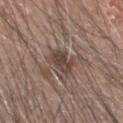| key | value |
|---|---|
| follow-up | total-body-photography surveillance lesion; no biopsy |
| subject | male, in their mid-40s |
| TBP lesion metrics | a lesion area of about 6.5 mm², an outline eccentricity of about 0.85 (0 = round, 1 = elongated), and a shape-asymmetry score of about 0.3 (0 = symmetric); a nevus-likeness score of about 15/100 and a detector confidence of about 90 out of 100 that the crop contains a lesion |
| image source | ~15 mm crop, total-body skin-cancer survey |
| illumination | white-light illumination |
| anatomic site | the head or neck |